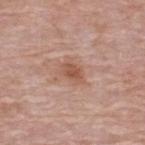site: the back
subject: male, in their mid- to late 60s
illumination: white-light
image source: ~15 mm crop, total-body skin-cancer survey
lesion diameter: ≈3 mm